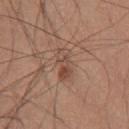The lesion was photographed on a routine skin check and not biopsied; there is no pathology result.
The lesion's longest dimension is about 4 mm.
The subject is a male roughly 60 years of age.
The lesion-visualizer software estimated an area of roughly 6.5 mm², an outline eccentricity of about 0.9 (0 = round, 1 = elongated), and a symmetry-axis asymmetry near 0.3. The software also gave a classifier nevus-likeness of about 80/100 and lesion-presence confidence of about 100/100.
Imaged with white-light lighting.
The lesion is on the chest.
Cropped from a total-body skin-imaging series; the visible field is about 15 mm.